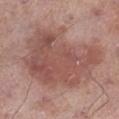Impression:
Imaged during a routine full-body skin examination; the lesion was not biopsied and no histopathology is available.
Background:
Cropped from a total-body skin-imaging series; the visible field is about 15 mm. A male subject, aged around 70. Captured under white-light illumination. Longest diameter approximately 10.5 mm. The lesion is on the leg.